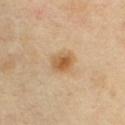workup = total-body-photography surveillance lesion; no biopsy
patient = male, aged 38–42
image source = ~15 mm crop, total-body skin-cancer survey
body site = the chest
illumination = cross-polarized illumination
lesion diameter = ≈2.5 mm